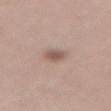<record>
<biopsy_status>not biopsied; imaged during a skin examination</biopsy_status>
<site>lower back</site>
<patient>
  <sex>female</sex>
  <age_approx>40</age_approx>
</patient>
<automated_metrics>
  <area_mm2_approx>3.5</area_mm2_approx>
  <eccentricity>0.8</eccentricity>
  <border_irregularity_0_10>2.5</border_irregularity_0_10>
  <peripheral_color_asymmetry>1.0</peripheral_color_asymmetry>
  <lesion_detection_confidence_0_100>100</lesion_detection_confidence_0_100>
</automated_metrics>
<image>
  <source>total-body photography crop</source>
  <field_of_view_mm>15</field_of_view_mm>
</image>
<lesion_size>
  <long_diameter_mm_approx>3.0</long_diameter_mm_approx>
</lesion_size>
</record>